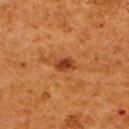Assessment: Imaged during a routine full-body skin examination; the lesion was not biopsied and no histopathology is available. Acquisition and patient details: From the upper back. The recorded lesion diameter is about 2.5 mm. A lesion tile, about 15 mm wide, cut from a 3D total-body photograph. A female subject roughly 50 years of age. The tile uses cross-polarized illumination. Automated tile analysis of the lesion measured an area of roughly 3.5 mm² and a symmetry-axis asymmetry near 0.25. And it measured an average lesion color of about L≈34 a*≈24 b*≈34 (CIELAB) and a lesion-to-skin contrast of about 8.5 (normalized; higher = more distinct). And it measured a border-irregularity rating of about 2/10 and a peripheral color-asymmetry measure near 0.5. The analysis additionally found a classifier nevus-likeness of about 65/100 and lesion-presence confidence of about 100/100.